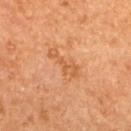Q: How was the tile lit?
A: cross-polarized
Q: Patient demographics?
A: female, roughly 60 years of age
Q: What is the imaging modality?
A: ~15 mm crop, total-body skin-cancer survey
Q: Automated lesion metrics?
A: an area of roughly 6.5 mm² and a shape eccentricity near 0.95; a border-irregularity rating of about 7.5/10, internal color variation of about 1.5 on a 0–10 scale, and radial color variation of about 0.5
Q: How large is the lesion?
A: about 5 mm
Q: Lesion location?
A: the upper back